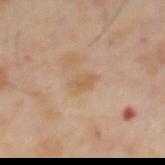<case>
<biopsy_status>not biopsied; imaged during a skin examination</biopsy_status>
<lesion_size>
  <long_diameter_mm_approx>2.5</long_diameter_mm_approx>
</lesion_size>
<automated_metrics>
  <area_mm2_approx>3.5</area_mm2_approx>
  <eccentricity>0.75</eccentricity>
  <border_irregularity_0_10>2.0</border_irregularity_0_10>
  <color_variation_0_10>1.0</color_variation_0_10>
  <nevus_likeness_0_100>0</nevus_likeness_0_100>
</automated_metrics>
<image>
  <source>total-body photography crop</source>
  <field_of_view_mm>15</field_of_view_mm>
</image>
<patient>
  <sex>male</sex>
  <age_approx>65</age_approx>
</patient>
<lighting>cross-polarized</lighting>
</case>